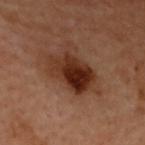Impression: This lesion was catalogued during total-body skin photography and was not selected for biopsy. Image and clinical context: A region of skin cropped from a whole-body photographic capture, roughly 15 mm wide. The total-body-photography lesion software estimated a lesion area of about 14 mm², an outline eccentricity of about 0.75 (0 = round, 1 = elongated), and a shape-asymmetry score of about 0.2 (0 = symmetric). The software also gave a mean CIELAB color near L≈25 a*≈20 b*≈25 and a lesion-to-skin contrast of about 12 (normalized; higher = more distinct). The subject is a female aged around 60. From the upper back. This is a cross-polarized tile. Approximately 5 mm at its widest.